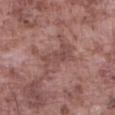{
  "biopsy_status": "not biopsied; imaged during a skin examination",
  "image": {
    "source": "total-body photography crop",
    "field_of_view_mm": 15
  },
  "site": "abdomen",
  "patient": {
    "sex": "male",
    "age_approx": 75
  },
  "automated_metrics": {
    "area_mm2_approx": 10.0,
    "shape_asymmetry": 0.4,
    "vs_skin_darker_L": 7.0,
    "vs_skin_contrast_norm": 5.5,
    "border_irregularity_0_10": 7.0,
    "color_variation_0_10": 3.0,
    "peripheral_color_asymmetry": 1.0
  },
  "lesion_size": {
    "long_diameter_mm_approx": 5.5
  }
}